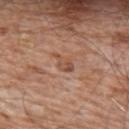Assessment:
Recorded during total-body skin imaging; not selected for excision or biopsy.
Acquisition and patient details:
Cropped from a whole-body photographic skin survey; the tile spans about 15 mm. The recorded lesion diameter is about 3 mm. A male subject, in their 80s. The lesion is on the upper back.